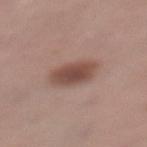Part of a total-body skin-imaging series; this lesion was reviewed on a skin check and was not flagged for biopsy. The patient is a female about 55 years old. A 15 mm close-up tile from a total-body photography series done for melanoma screening. On the left lower leg. About 4 mm across. An algorithmic analysis of the crop reported an automated nevus-likeness rating near 100 out of 100. This is a white-light tile.